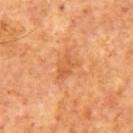The lesion was tiled from a total-body skin photograph and was not biopsied. This is a cross-polarized tile. The subject is a male in their mid-60s. Approximately 4.5 mm at its widest. A 15 mm close-up tile from a total-body photography series done for melanoma screening.